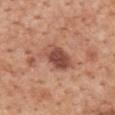<case>
  <biopsy_status>not biopsied; imaged during a skin examination</biopsy_status>
</case>